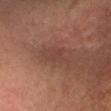A 15 mm close-up tile from a total-body photography series done for melanoma screening.
Measured at roughly 4 mm in maximum diameter.
Automated tile analysis of the lesion measured a footprint of about 6.5 mm², an outline eccentricity of about 0.8 (0 = round, 1 = elongated), and two-axis asymmetry of about 0.35. And it measured a border-irregularity index near 4/10 and peripheral color asymmetry of about 0.5. The software also gave an automated nevus-likeness rating near 0 out of 100 and a lesion-detection confidence of about 95/100.
The lesion is located on the head or neck.
The subject is a female roughly 45 years of age.